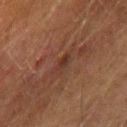No biopsy was performed on this lesion — it was imaged during a full skin examination and was not determined to be concerning. The total-body-photography lesion software estimated a lesion area of about 4 mm², an eccentricity of roughly 0.9, and a symmetry-axis asymmetry near 0.3. The analysis additionally found roughly 5 lightness units darker than nearby skin and a normalized lesion–skin contrast near 5.5. And it measured a classifier nevus-likeness of about 0/100 and lesion-presence confidence of about 70/100. Cropped from a whole-body photographic skin survey; the tile spans about 15 mm. Captured under cross-polarized illumination. A male patient aged around 75. Approximately 3 mm at its widest. Located on the right upper arm.